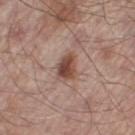- notes: no biopsy performed (imaged during a skin exam)
- illumination: white-light
- subject: male, in their mid- to late 50s
- image source: 15 mm crop, total-body photography
- body site: the leg
- TBP lesion metrics: a lesion–skin lightness drop of about 12 and a lesion-to-skin contrast of about 9 (normalized; higher = more distinct); lesion-presence confidence of about 100/100
- lesion diameter: about 3.5 mm